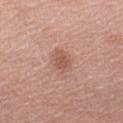Q: Was this lesion biopsied?
A: no biopsy performed (imaged during a skin exam)
Q: How was this image acquired?
A: 15 mm crop, total-body photography
Q: Automated lesion metrics?
A: border irregularity of about 2.5 on a 0–10 scale, a color-variation rating of about 1.5/10, and a peripheral color-asymmetry measure near 0.5; a classifier nevus-likeness of about 50/100 and a lesion-detection confidence of about 100/100
Q: Lesion location?
A: the right upper arm
Q: Patient demographics?
A: female, aged around 65
Q: How was the tile lit?
A: white-light